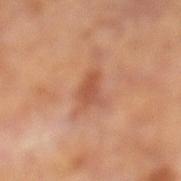Assessment: Captured during whole-body skin photography for melanoma surveillance; the lesion was not biopsied. Background: A male subject, about 65 years old. On the left lower leg. Approximately 3 mm at its widest. Imaged with cross-polarized lighting. A region of skin cropped from a whole-body photographic capture, roughly 15 mm wide.